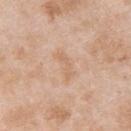{"automated_metrics": {"area_mm2_approx": 2.5, "eccentricity": 0.95, "shape_asymmetry": 0.6, "cielab_L": 65, "cielab_a": 18, "cielab_b": 33, "vs_skin_contrast_norm": 4.5}, "lighting": "white-light", "image": {"source": "total-body photography crop", "field_of_view_mm": 15}, "patient": {"sex": "male", "age_approx": 25}, "site": "upper back"}